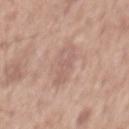Case summary:
• notes — imaged on a skin check; not biopsied
• anatomic site — the mid back
• image — ~15 mm tile from a whole-body skin photo
• patient — male, aged 58 to 62